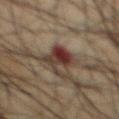– illumination: cross-polarized illumination
– image source: 15 mm crop, total-body photography
– body site: the chest
– subject: male, aged approximately 65
– size: ~5.5 mm (longest diameter)